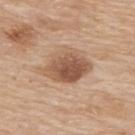The lesion was photographed on a routine skin check and not biopsied; there is no pathology result. The lesion is on the upper back. A roughly 15 mm field-of-view crop from a total-body skin photograph. The lesion-visualizer software estimated a lesion area of about 14 mm². It also reported a mean CIELAB color near L≈54 a*≈20 b*≈31, a lesion–skin lightness drop of about 13, and a normalized lesion–skin contrast near 9. The software also gave a border-irregularity rating of about 2/10. It also reported a nevus-likeness score of about 80/100 and lesion-presence confidence of about 100/100. A male subject approximately 80 years of age. The recorded lesion diameter is about 5.5 mm.